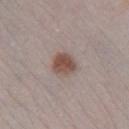Q: Is there a histopathology result?
A: imaged on a skin check; not biopsied
Q: Lesion size?
A: ≈3 mm
Q: How was this image acquired?
A: total-body-photography crop, ~15 mm field of view
Q: What is the anatomic site?
A: the right lower leg
Q: Illumination type?
A: white-light illumination
Q: What are the patient's age and sex?
A: female, approximately 30 years of age